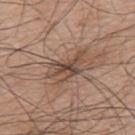subject: male, aged 73–77 | image: ~15 mm crop, total-body skin-cancer survey | tile lighting: white-light | anatomic site: the back | lesion diameter: ≈5.5 mm | image-analysis metrics: a mean CIELAB color near L≈47 a*≈16 b*≈26, roughly 10 lightness units darker than nearby skin, and a lesion-to-skin contrast of about 8 (normalized; higher = more distinct); internal color variation of about 6 on a 0–10 scale and radial color variation of about 1.5; a classifier nevus-likeness of about 0/100 and lesion-presence confidence of about 65/100.